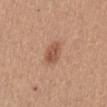Assessment:
No biopsy was performed on this lesion — it was imaged during a full skin examination and was not determined to be concerning.
Acquisition and patient details:
The patient is a female in their mid- to late 50s. An algorithmic analysis of the crop reported an area of roughly 5.5 mm² and a shape-asymmetry score of about 0.3 (0 = symmetric). The software also gave an average lesion color of about L≈53 a*≈23 b*≈31 (CIELAB) and roughly 10 lightness units darker than nearby skin. A 15 mm close-up extracted from a 3D total-body photography capture. The lesion is on the abdomen.